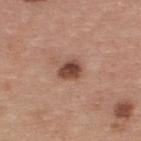notes: imaged on a skin check; not biopsied
body site: the upper back
subject: male, in their mid-30s
lighting: white-light illumination
image: ~15 mm tile from a whole-body skin photo